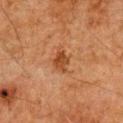{
  "lighting": "cross-polarized",
  "site": "chest",
  "patient": {
    "sex": "male",
    "age_approx": 80
  },
  "image": {
    "source": "total-body photography crop",
    "field_of_view_mm": 15
  },
  "automated_metrics": {
    "area_mm2_approx": 4.0,
    "eccentricity": 0.65,
    "shape_asymmetry": 0.25,
    "border_irregularity_0_10": 2.5,
    "color_variation_0_10": 1.5,
    "nevus_likeness_0_100": 70,
    "lesion_detection_confidence_0_100": 100
  }
}